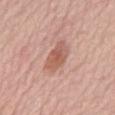Q: Illumination type?
A: white-light illumination
Q: Lesion location?
A: the mid back
Q: What kind of image is this?
A: 15 mm crop, total-body photography
Q: How large is the lesion?
A: ≈4 mm
Q: Patient demographics?
A: female, approximately 65 years of age
Q: What did automated image analysis measure?
A: a border-irregularity rating of about 2.5/10, a within-lesion color-variation index near 4.5/10, and a peripheral color-asymmetry measure near 1.5; a classifier nevus-likeness of about 45/100 and a lesion-detection confidence of about 100/100